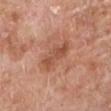anatomic site = the left lower leg; subject = male, aged 78 to 82; lesion size = about 5 mm; image = total-body-photography crop, ~15 mm field of view.